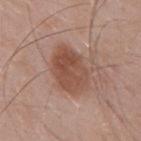biopsy_status: not biopsied; imaged during a skin examination
patient:
  sex: male
  age_approx: 55
site: mid back
lighting: white-light
image:
  source: total-body photography crop
  field_of_view_mm: 15